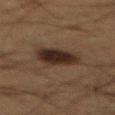workup=no biopsy performed (imaged during a skin exam)
subject=male, aged approximately 65
tile lighting=cross-polarized illumination
image=total-body-photography crop, ~15 mm field of view
body site=the mid back